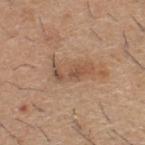Assessment: No biopsy was performed on this lesion — it was imaged during a full skin examination and was not determined to be concerning. Background: A male subject, aged approximately 60. The recorded lesion diameter is about 4.5 mm. The lesion is on the upper back. A 15 mm crop from a total-body photograph taken for skin-cancer surveillance. This is a white-light tile.